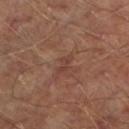Imaged during a routine full-body skin examination; the lesion was not biopsied and no histopathology is available.
A male subject, roughly 65 years of age.
The lesion is located on the left lower leg.
This image is a 15 mm lesion crop taken from a total-body photograph.
This is a cross-polarized tile.
About 2.5 mm across.